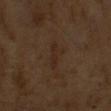Impression:
Part of a total-body skin-imaging series; this lesion was reviewed on a skin check and was not flagged for biopsy.
Image and clinical context:
Imaged with cross-polarized lighting. A male subject aged approximately 65. A region of skin cropped from a whole-body photographic capture, roughly 15 mm wide.